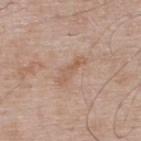Q: Is there a histopathology result?
A: imaged on a skin check; not biopsied
Q: How was the tile lit?
A: white-light
Q: What kind of image is this?
A: total-body-photography crop, ~15 mm field of view
Q: Who is the patient?
A: male, aged approximately 65
Q: Lesion location?
A: the upper back
Q: What did automated image analysis measure?
A: an area of roughly 5.5 mm², an eccentricity of roughly 0.95, and a symmetry-axis asymmetry near 0.3; an automated nevus-likeness rating near 0 out of 100 and a detector confidence of about 100 out of 100 that the crop contains a lesion
Q: How large is the lesion?
A: ≈4 mm